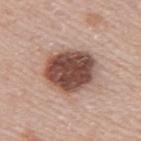Part of a total-body skin-imaging series; this lesion was reviewed on a skin check and was not flagged for biopsy. This is a white-light tile. The lesion is located on the right upper arm. The patient is a female aged around 50. Automated image analysis of the tile measured a footprint of about 21 mm² and an eccentricity of roughly 0.6. The software also gave an automated nevus-likeness rating near 30 out of 100 and lesion-presence confidence of about 100/100. A 15 mm close-up tile from a total-body photography series done for melanoma screening. Measured at roughly 6 mm in maximum diameter.